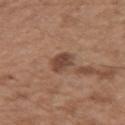Q: Who is the patient?
A: male, aged 53 to 57
Q: What is the anatomic site?
A: the left upper arm
Q: How was this image acquired?
A: total-body-photography crop, ~15 mm field of view